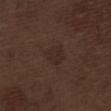No biopsy was performed on this lesion — it was imaged during a full skin examination and was not determined to be concerning. Automated tile analysis of the lesion measured an area of roughly 5.5 mm², a shape eccentricity near 0.4, and a shape-asymmetry score of about 0.15 (0 = symmetric). The software also gave a normalized border contrast of about 4.5. It also reported border irregularity of about 1.5 on a 0–10 scale and a peripheral color-asymmetry measure near 0.5. The subject is a male roughly 70 years of age. On the leg. A lesion tile, about 15 mm wide, cut from a 3D total-body photograph.